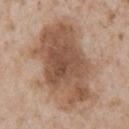No biopsy was performed on this lesion — it was imaged during a full skin examination and was not determined to be concerning.